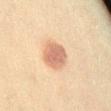The lesion was tiled from a total-body skin photograph and was not biopsied.
A female subject, about 40 years old.
The lesion is located on the abdomen.
This is a cross-polarized tile.
A close-up tile cropped from a whole-body skin photograph, about 15 mm across.